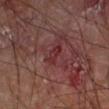Q: Was this lesion biopsied?
A: no biopsy performed (imaged during a skin exam)
Q: Where on the body is the lesion?
A: the left forearm
Q: What are the patient's age and sex?
A: male, roughly 60 years of age
Q: Automated lesion metrics?
A: a classifier nevus-likeness of about 0/100
Q: How was this image acquired?
A: total-body-photography crop, ~15 mm field of view
Q: What lighting was used for the tile?
A: cross-polarized
Q: How large is the lesion?
A: ≈2.5 mm